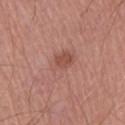Clinical impression: No biopsy was performed on this lesion — it was imaged during a full skin examination and was not determined to be concerning. Context: The total-body-photography lesion software estimated an area of roughly 8.5 mm² and an eccentricity of roughly 0.6. And it measured a lesion–skin lightness drop of about 7. The software also gave a classifier nevus-likeness of about 70/100. A close-up tile cropped from a whole-body skin photograph, about 15 mm across. On the right upper arm. Imaged with white-light lighting. A male subject, aged 63 to 67.